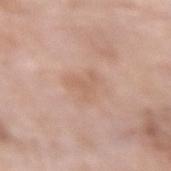Imaged during a routine full-body skin examination; the lesion was not biopsied and no histopathology is available. Automated image analysis of the tile measured a lesion area of about 6 mm² and an outline eccentricity of about 0.5 (0 = round, 1 = elongated). The analysis additionally found a nevus-likeness score of about 0/100 and a detector confidence of about 100 out of 100 that the crop contains a lesion. From the left forearm. Measured at roughly 3 mm in maximum diameter. Cropped from a whole-body photographic skin survey; the tile spans about 15 mm. A female patient aged approximately 75.